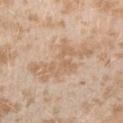On the arm.
Longest diameter approximately 8 mm.
The patient is a female in their mid-20s.
Imaged with white-light lighting.
Automated tile analysis of the lesion measured an area of roughly 19 mm², an eccentricity of roughly 0.9, and two-axis asymmetry of about 0.45. The analysis additionally found a lesion–skin lightness drop of about 7 and a normalized border contrast of about 5.5. The analysis additionally found a nevus-likeness score of about 0/100.
Cropped from a whole-body photographic skin survey; the tile spans about 15 mm.